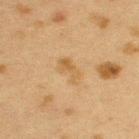Notes:
* notes · imaged on a skin check; not biopsied
* subject · female, approximately 40 years of age
* site · the left upper arm
* illumination · cross-polarized
* acquisition · ~15 mm crop, total-body skin-cancer survey
* diameter · ≈2.5 mm